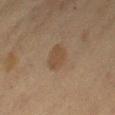biopsy status: imaged on a skin check; not biopsied
image-analysis metrics: an outline eccentricity of about 0.75 (0 = round, 1 = elongated) and a shape-asymmetry score of about 0.2 (0 = symmetric)
patient: female, aged approximately 40
image source: 15 mm crop, total-body photography
site: the leg